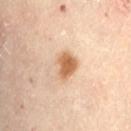The lesion is on the back. Cropped from a whole-body photographic skin survey; the tile spans about 15 mm. The recorded lesion diameter is about 4 mm. Automated image analysis of the tile measured a mean CIELAB color near L≈64 a*≈20 b*≈37, a lesion–skin lightness drop of about 14, and a normalized border contrast of about 9. It also reported a classifier nevus-likeness of about 100/100 and a lesion-detection confidence of about 100/100. The tile uses cross-polarized illumination. The subject is a female aged around 60.